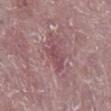{
  "biopsy_status": "not biopsied; imaged during a skin examination",
  "image": {
    "source": "total-body photography crop",
    "field_of_view_mm": 15
  },
  "automated_metrics": {
    "cielab_L": 48,
    "cielab_a": 25,
    "cielab_b": 15,
    "vs_skin_darker_L": 8.0,
    "vs_skin_contrast_norm": 6.0,
    "color_variation_0_10": 2.5,
    "peripheral_color_asymmetry": 1.0,
    "nevus_likeness_0_100": 0,
    "lesion_detection_confidence_0_100": 90
  },
  "patient": {
    "sex": "male",
    "age_approx": 75
  },
  "site": "right lower leg",
  "lesion_size": {
    "long_diameter_mm_approx": 3.5
  }
}